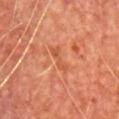Q: Was this lesion biopsied?
A: no biopsy performed (imaged during a skin exam)
Q: What is the imaging modality?
A: 15 mm crop, total-body photography
Q: What lighting was used for the tile?
A: cross-polarized illumination
Q: What is the anatomic site?
A: the chest
Q: Patient demographics?
A: male, aged approximately 65
Q: How large is the lesion?
A: ~3 mm (longest diameter)